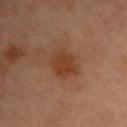{"biopsy_status": "not biopsied; imaged during a skin examination", "automated_metrics": {"area_mm2_approx": 9.0, "eccentricity": 0.65, "shape_asymmetry": 0.2, "cielab_L": 32, "cielab_a": 18, "cielab_b": 27, "vs_skin_darker_L": 6.0, "border_irregularity_0_10": 2.0, "color_variation_0_10": 2.5, "peripheral_color_asymmetry": 1.0, "nevus_likeness_0_100": 65, "lesion_detection_confidence_0_100": 100}, "lighting": "cross-polarized", "site": "chest", "patient": {"sex": "female", "age_approx": 55}, "image": {"source": "total-body photography crop", "field_of_view_mm": 15}, "lesion_size": {"long_diameter_mm_approx": 4.0}}